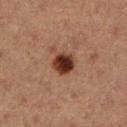workup: catalogued during a skin exam; not biopsied | image source: ~15 mm crop, total-body skin-cancer survey | subject: female, in their 40s | location: the left lower leg.